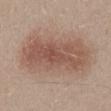No biopsy was performed on this lesion — it was imaged during a full skin examination and was not determined to be concerning. Captured under white-light illumination. About 9.5 mm across. A close-up tile cropped from a whole-body skin photograph, about 15 mm across. Located on the back. The subject is a male aged 28–32. The lesion-visualizer software estimated a mean CIELAB color near L≈54 a*≈18 b*≈26, about 10 CIELAB-L* units darker than the surrounding skin, and a normalized lesion–skin contrast near 7. The software also gave a border-irregularity index near 3.5/10, internal color variation of about 5 on a 0–10 scale, and a peripheral color-asymmetry measure near 1.5.